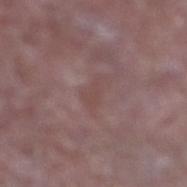A roughly 15 mm field-of-view crop from a total-body skin photograph.
The patient is a male aged approximately 65.
The lesion's longest dimension is about 3 mm.
From the right lower leg.
Captured under white-light illumination.